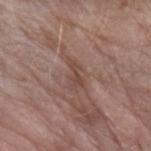This lesion was catalogued during total-body skin photography and was not selected for biopsy. The lesion-visualizer software estimated an outline eccentricity of about 0.9 (0 = round, 1 = elongated) and two-axis asymmetry of about 0.5. It also reported a border-irregularity index near 6/10, a color-variation rating of about 0/10, and a peripheral color-asymmetry measure near 0. The software also gave a nevus-likeness score of about 0/100. The lesion is on the left forearm. This is a white-light tile. A female subject approximately 75 years of age. Longest diameter approximately 3 mm. A lesion tile, about 15 mm wide, cut from a 3D total-body photograph.